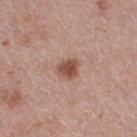Notes:
- biopsy status — no biopsy performed (imaged during a skin exam)
- lighting — white-light
- TBP lesion metrics — a border-irregularity index near 2/10 and a within-lesion color-variation index near 3/10; a classifier nevus-likeness of about 95/100 and lesion-presence confidence of about 100/100
- anatomic site — the right thigh
- patient — female, aged around 40
- acquisition — total-body-photography crop, ~15 mm field of view